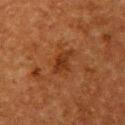No biopsy was performed on this lesion — it was imaged during a full skin examination and was not determined to be concerning. The total-body-photography lesion software estimated a footprint of about 4.5 mm², an eccentricity of roughly 0.85, and two-axis asymmetry of about 0.4. And it measured a peripheral color-asymmetry measure near 0.5. And it measured an automated nevus-likeness rating near 0 out of 100 and lesion-presence confidence of about 100/100. Measured at roughly 3 mm in maximum diameter. A lesion tile, about 15 mm wide, cut from a 3D total-body photograph. From the arm. A female subject, aged approximately 50.